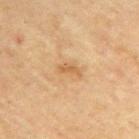Assessment: This lesion was catalogued during total-body skin photography and was not selected for biopsy. Clinical summary: The lesion's longest dimension is about 3 mm. An algorithmic analysis of the crop reported an eccentricity of roughly 0.9 and a symmetry-axis asymmetry near 0.45. The analysis additionally found a lesion–skin lightness drop of about 8. The software also gave a border-irregularity rating of about 5/10, a within-lesion color-variation index near 0/10, and a peripheral color-asymmetry measure near 0. Captured under cross-polarized illumination. The lesion is located on the right upper arm. A close-up tile cropped from a whole-body skin photograph, about 15 mm across. A male subject roughly 45 years of age.